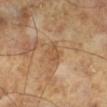image-analysis metrics: a footprint of about 6 mm², an outline eccentricity of about 0.65 (0 = round, 1 = elongated), and a symmetry-axis asymmetry near 0.25; a lesion color around L≈52 a*≈18 b*≈34 in CIELAB and a lesion-to-skin contrast of about 5.5 (normalized; higher = more distinct); a classifier nevus-likeness of about 0/100 and a lesion-detection confidence of about 100/100 | subject: male, aged around 65 | imaging modality: total-body-photography crop, ~15 mm field of view | tile lighting: cross-polarized illumination | lesion diameter: ~3 mm (longest diameter).